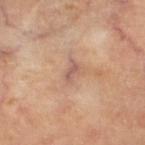Part of a total-body skin-imaging series; this lesion was reviewed on a skin check and was not flagged for biopsy. A region of skin cropped from a whole-body photographic capture, roughly 15 mm wide. A patient aged around 60. The lesion is on the left lower leg. Imaged with cross-polarized lighting. About 2.5 mm across.